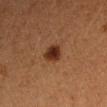Imaged during a routine full-body skin examination; the lesion was not biopsied and no histopathology is available. The total-body-photography lesion software estimated a lesion–skin lightness drop of about 11 and a lesion-to-skin contrast of about 11.5 (normalized; higher = more distinct). The software also gave a nevus-likeness score of about 100/100 and a detector confidence of about 100 out of 100 that the crop contains a lesion. A female patient aged 38–42. Measured at roughly 2.5 mm in maximum diameter. A close-up tile cropped from a whole-body skin photograph, about 15 mm across. On the left upper arm.